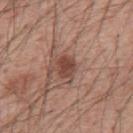Q: What is the anatomic site?
A: the mid back
Q: What did automated image analysis measure?
A: a footprint of about 5 mm² and an outline eccentricity of about 0.65 (0 = round, 1 = elongated); a color-variation rating of about 2.5/10 and a peripheral color-asymmetry measure near 1; a nevus-likeness score of about 90/100 and a lesion-detection confidence of about 100/100
Q: How was this image acquired?
A: 15 mm crop, total-body photography
Q: Who is the patient?
A: male, aged 53 to 57